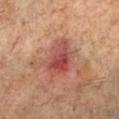Clinical summary:
The recorded lesion diameter is about 5 mm. The lesion-visualizer software estimated an area of roughly 12 mm², a shape eccentricity near 0.75, and a shape-asymmetry score of about 0.25 (0 = symmetric). The software also gave a mean CIELAB color near L≈46 a*≈28 b*≈25, about 10 CIELAB-L* units darker than the surrounding skin, and a normalized lesion–skin contrast near 8. A 15 mm crop from a total-body photograph taken for skin-cancer surveillance. From the left lower leg. The patient is a male aged 58–62. Captured under cross-polarized illumination.